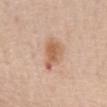Context:
A female subject, aged 68 to 72. Located on the chest. The total-body-photography lesion software estimated an eccentricity of roughly 0.7 and a shape-asymmetry score of about 0.3 (0 = symmetric). It also reported a border-irregularity index near 3/10 and a peripheral color-asymmetry measure near 1. This image is a 15 mm lesion crop taken from a total-body photograph. The recorded lesion diameter is about 4 mm.